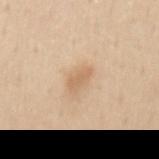Imaged during a routine full-body skin examination; the lesion was not biopsied and no histopathology is available.
The recorded lesion diameter is about 2.5 mm.
This image is a 15 mm lesion crop taken from a total-body photograph.
The lesion-visualizer software estimated an area of roughly 3 mm². It also reported a color-variation rating of about 1/10 and radial color variation of about 0.5. The analysis additionally found a nevus-likeness score of about 45/100 and a detector confidence of about 100 out of 100 that the crop contains a lesion.
Located on the mid back.
Imaged with white-light lighting.
A female subject in their mid- to late 40s.